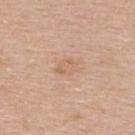The lesion was tiled from a total-body skin photograph and was not biopsied.
The patient is a male aged around 35.
The recorded lesion diameter is about 3 mm.
The tile uses white-light illumination.
From the upper back.
This image is a 15 mm lesion crop taken from a total-body photograph.
Automated tile analysis of the lesion measured an automated nevus-likeness rating near 0 out of 100 and a lesion-detection confidence of about 100/100.